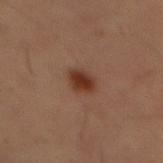Captured during whole-body skin photography for melanoma surveillance; the lesion was not biopsied. From the abdomen. A close-up tile cropped from a whole-body skin photograph, about 15 mm across. Imaged with cross-polarized lighting. A male patient, aged around 55.